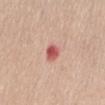Impression: The lesion was tiled from a total-body skin photograph and was not biopsied. Acquisition and patient details: Cropped from a total-body skin-imaging series; the visible field is about 15 mm. A female subject approximately 45 years of age. From the abdomen.